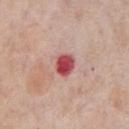Q: Was a biopsy performed?
A: total-body-photography surveillance lesion; no biopsy
Q: Where on the body is the lesion?
A: the chest
Q: What lighting was used for the tile?
A: white-light
Q: Automated lesion metrics?
A: a footprint of about 6 mm² and an eccentricity of roughly 0.65; an average lesion color of about L≈51 a*≈35 b*≈23 (CIELAB), roughly 17 lightness units darker than nearby skin, and a normalized border contrast of about 11
Q: How was this image acquired?
A: ~15 mm crop, total-body skin-cancer survey
Q: How large is the lesion?
A: ≈3 mm
Q: Who is the patient?
A: male, approximately 75 years of age